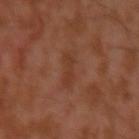| feature | finding |
|---|---|
| biopsy status | no biopsy performed (imaged during a skin exam) |
| tile lighting | cross-polarized illumination |
| subject | male, roughly 30 years of age |
| imaging modality | total-body-photography crop, ~15 mm field of view |
| lesion size | about 3.5 mm |
| site | the left upper arm |